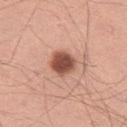Captured during whole-body skin photography for melanoma surveillance; the lesion was not biopsied.
The subject is a male aged around 30.
About 3 mm across.
A close-up tile cropped from a whole-body skin photograph, about 15 mm across.
The lesion is located on the left thigh.
Captured under white-light illumination.